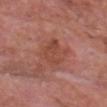biopsy status: imaged on a skin check; not biopsied
subject: male, approximately 60 years of age
diameter: ≈4 mm
acquisition: 15 mm crop, total-body photography
body site: the chest